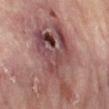| field | value |
|---|---|
| follow-up | total-body-photography surveillance lesion; no biopsy |
| lighting | cross-polarized illumination |
| site | the right lower leg |
| image source | 15 mm crop, total-body photography |
| patient | female, aged 73 to 77 |
| image-analysis metrics | a footprint of about 40 mm², an outline eccentricity of about 0.85 (0 = round, 1 = elongated), and a shape-asymmetry score of about 0.45 (0 = symmetric); a lesion color around L≈47 a*≈22 b*≈20 in CIELAB |